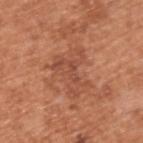Clinical impression:
Part of a total-body skin-imaging series; this lesion was reviewed on a skin check and was not flagged for biopsy.
Background:
Longest diameter approximately 5 mm. The tile uses white-light illumination. The lesion is located on the upper back. A region of skin cropped from a whole-body photographic capture, roughly 15 mm wide. Automated tile analysis of the lesion measured a footprint of about 11 mm², a shape eccentricity near 0.7, and two-axis asymmetry of about 0.65. It also reported an average lesion color of about L≈50 a*≈26 b*≈33 (CIELAB), about 7 CIELAB-L* units darker than the surrounding skin, and a normalized lesion–skin contrast near 5.5. A male patient, aged 53 to 57.